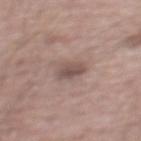No biopsy was performed on this lesion — it was imaged during a full skin examination and was not determined to be concerning.
A close-up tile cropped from a whole-body skin photograph, about 15 mm across.
The total-body-photography lesion software estimated an area of roughly 5 mm² and an eccentricity of roughly 0.5.
From the mid back.
Measured at roughly 2.5 mm in maximum diameter.
A male subject, aged around 55.